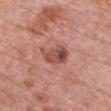Longest diameter approximately 4 mm. The lesion is located on the chest. Cropped from a total-body skin-imaging series; the visible field is about 15 mm. A female subject, in their 60s.